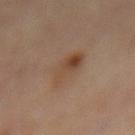notes: no biopsy performed (imaged during a skin exam) | image source: 15 mm crop, total-body photography | illumination: cross-polarized illumination | body site: the back | subject: male, about 65 years old | lesion diameter: ≈4.5 mm | image-analysis metrics: a footprint of about 10 mm², an outline eccentricity of about 0.7 (0 = round, 1 = elongated), and two-axis asymmetry of about 0.2; a lesion color around L≈43 a*≈16 b*≈28 in CIELAB, roughly 7 lightness units darker than nearby skin, and a normalized border contrast of about 6.5; a border-irregularity rating of about 2.5/10, internal color variation of about 7.5 on a 0–10 scale, and peripheral color asymmetry of about 2.5; an automated nevus-likeness rating near 60 out of 100.